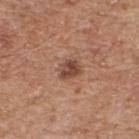This lesion was catalogued during total-body skin photography and was not selected for biopsy. Automated image analysis of the tile measured a lesion area of about 4.5 mm², an eccentricity of roughly 0.6, and two-axis asymmetry of about 0.25. It also reported an automated nevus-likeness rating near 55 out of 100. A close-up tile cropped from a whole-body skin photograph, about 15 mm across. A male patient, roughly 65 years of age. On the upper back. About 3 mm across. Imaged with white-light lighting.